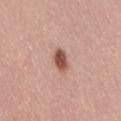Q: Is there a histopathology result?
A: no biopsy performed (imaged during a skin exam)
Q: Illumination type?
A: white-light illumination
Q: How was this image acquired?
A: ~15 mm tile from a whole-body skin photo
Q: What are the patient's age and sex?
A: female, aged around 40
Q: What is the anatomic site?
A: the back